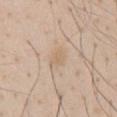Recorded during total-body skin imaging; not selected for excision or biopsy.
From the chest.
A region of skin cropped from a whole-body photographic capture, roughly 15 mm wide.
Longest diameter approximately 2.5 mm.
Captured under white-light illumination.
A male patient roughly 60 years of age.
The total-body-photography lesion software estimated an area of roughly 4 mm² and an outline eccentricity of about 0.65 (0 = round, 1 = elongated). And it measured an average lesion color of about L≈65 a*≈14 b*≈31 (CIELAB), about 5 CIELAB-L* units darker than the surrounding skin, and a normalized lesion–skin contrast near 4.5. The analysis additionally found internal color variation of about 1.5 on a 0–10 scale and a peripheral color-asymmetry measure near 0.5. The software also gave a classifier nevus-likeness of about 0/100.